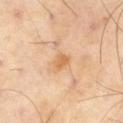Q: Was a biopsy performed?
A: imaged on a skin check; not biopsied
Q: What is the anatomic site?
A: the right thigh
Q: Lesion size?
A: about 2.5 mm
Q: How was this image acquired?
A: total-body-photography crop, ~15 mm field of view
Q: Illumination type?
A: cross-polarized
Q: What did automated image analysis measure?
A: a shape eccentricity near 0.55 and two-axis asymmetry of about 0.35; a lesion color around L≈67 a*≈21 b*≈41 in CIELAB, a lesion–skin lightness drop of about 8, and a lesion-to-skin contrast of about 6.5 (normalized; higher = more distinct); a detector confidence of about 100 out of 100 that the crop contains a lesion
Q: What are the patient's age and sex?
A: male, approximately 55 years of age